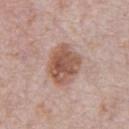Imaged during a routine full-body skin examination; the lesion was not biopsied and no histopathology is available.
This is a white-light tile.
The total-body-photography lesion software estimated a lesion area of about 17 mm², an eccentricity of roughly 0.55, and two-axis asymmetry of about 0.15. And it measured a lesion color around L≈55 a*≈20 b*≈27 in CIELAB, a lesion–skin lightness drop of about 12, and a lesion-to-skin contrast of about 8.5 (normalized; higher = more distinct). It also reported a within-lesion color-variation index near 5/10 and a peripheral color-asymmetry measure near 1.5.
The lesion is on the chest.
Longest diameter approximately 5 mm.
A 15 mm close-up tile from a total-body photography series done for melanoma screening.
A male patient, aged 68 to 72.